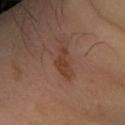The lesion was photographed on a routine skin check and not biopsied; there is no pathology result.
The subject is a male aged approximately 65.
The lesion is on the right forearm.
This image is a 15 mm lesion crop taken from a total-body photograph.
This is a cross-polarized tile.
Approximately 3.5 mm at its widest.